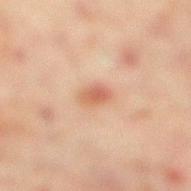notes: total-body-photography surveillance lesion; no biopsy
acquisition: 15 mm crop, total-body photography
automated metrics: a detector confidence of about 100 out of 100 that the crop contains a lesion
anatomic site: the right lower leg
subject: male, in their mid- to late 40s
illumination: cross-polarized illumination
lesion size: ~2.5 mm (longest diameter)